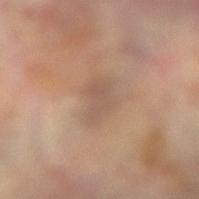| field | value |
|---|---|
| follow-up | imaged on a skin check; not biopsied |
| location | the right forearm |
| image source | 15 mm crop, total-body photography |
| subject | female, aged 73–77 |